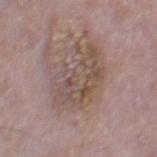Assessment: Captured during whole-body skin photography for melanoma surveillance; the lesion was not biopsied. Image and clinical context: The recorded lesion diameter is about 6.5 mm. Cropped from a total-body skin-imaging series; the visible field is about 15 mm. From the right thigh. A male patient, approximately 65 years of age. Captured under white-light illumination.